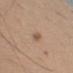Notes:
– workup — catalogued during a skin exam; not biopsied
– patient — male, aged 58–62
– lesion diameter — ≈3 mm
– illumination — white-light illumination
– automated metrics — an eccentricity of roughly 0.95 and a shape-asymmetry score of about 0.4 (0 = symmetric); a classifier nevus-likeness of about 0/100
– location — the right upper arm
– image — 15 mm crop, total-body photography